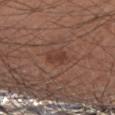Recorded during total-body skin imaging; not selected for excision or biopsy.
A male subject roughly 60 years of age.
The lesion is on the arm.
A lesion tile, about 15 mm wide, cut from a 3D total-body photograph.
Approximately 2 mm at its widest.